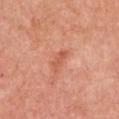follow-up = no biopsy performed (imaged during a skin exam); lesion diameter = about 3 mm; imaging modality = total-body-photography crop, ~15 mm field of view; body site = the chest; TBP lesion metrics = an average lesion color of about L≈58 a*≈31 b*≈35 (CIELAB), roughly 8 lightness units darker than nearby skin, and a normalized lesion–skin contrast near 6; patient = female, approximately 55 years of age.